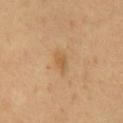workup — total-body-photography surveillance lesion; no biopsy | body site — the front of the torso | subject — male, in their mid- to late 60s | image-analysis metrics — a mean CIELAB color near L≈57 a*≈19 b*≈39, about 8 CIELAB-L* units darker than the surrounding skin, and a lesion-to-skin contrast of about 6 (normalized; higher = more distinct) | size — ~3 mm (longest diameter) | tile lighting — cross-polarized illumination | image — ~15 mm tile from a whole-body skin photo.